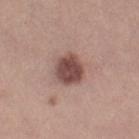{
  "biopsy_status": "not biopsied; imaged during a skin examination"
}